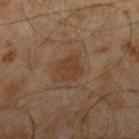Imaged during a routine full-body skin examination; the lesion was not biopsied and no histopathology is available. Located on the right lower leg. The lesion's longest dimension is about 3 mm. A male subject aged approximately 45. A 15 mm close-up extracted from a 3D total-body photography capture.